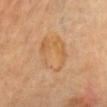Assessment:
Imaged during a routine full-body skin examination; the lesion was not biopsied and no histopathology is available.
Image and clinical context:
An algorithmic analysis of the crop reported a footprint of about 15 mm², an eccentricity of roughly 0.55, and a shape-asymmetry score of about 0.15 (0 = symmetric). And it measured a border-irregularity index near 1.5/10, a color-variation rating of about 5/10, and radial color variation of about 1.5. A male patient, approximately 70 years of age. A close-up tile cropped from a whole-body skin photograph, about 15 mm across. The lesion's longest dimension is about 5 mm. The tile uses cross-polarized illumination. The lesion is on the chest.